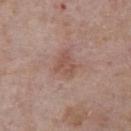biopsy status=no biopsy performed (imaged during a skin exam) | site=the chest | automated lesion analysis=an average lesion color of about L≈52 a*≈20 b*≈26 (CIELAB), about 8 CIELAB-L* units darker than the surrounding skin, and a normalized lesion–skin contrast near 6; border irregularity of about 4 on a 0–10 scale; a classifier nevus-likeness of about 0/100 | acquisition=total-body-photography crop, ~15 mm field of view | subject=male, aged 73 to 77.